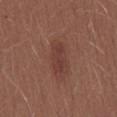The lesion was tiled from a total-body skin photograph and was not biopsied. The total-body-photography lesion software estimated a shape-asymmetry score of about 0.25 (0 = symmetric). The analysis additionally found an average lesion color of about L≈39 a*≈22 b*≈24 (CIELAB), about 7 CIELAB-L* units darker than the surrounding skin, and a lesion-to-skin contrast of about 6 (normalized; higher = more distinct). The software also gave border irregularity of about 3 on a 0–10 scale, a within-lesion color-variation index near 2/10, and peripheral color asymmetry of about 0.5. And it measured a lesion-detection confidence of about 100/100. On the mid back. A female subject, roughly 25 years of age. Captured under white-light illumination. Longest diameter approximately 4 mm. Cropped from a total-body skin-imaging series; the visible field is about 15 mm.